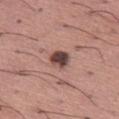follow-up = imaged on a skin check; not biopsied
image source = ~15 mm crop, total-body skin-cancer survey
illumination = white-light
anatomic site = the left thigh
size = about 2.5 mm
subject = male, aged approximately 45